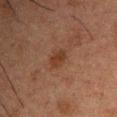workup = imaged on a skin check; not biopsied | patient = male, aged 48 to 52 | imaging modality = ~15 mm tile from a whole-body skin photo | lesion size = about 2.5 mm | lighting = cross-polarized | anatomic site = the left upper arm.